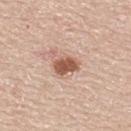The tile uses white-light illumination.
The lesion is located on the upper back.
An algorithmic analysis of the crop reported an area of roughly 5.5 mm² and a shape eccentricity near 0.75. And it measured a border-irregularity index near 2/10, a color-variation rating of about 2.5/10, and a peripheral color-asymmetry measure near 1.
A male subject, about 60 years old.
Longest diameter approximately 3 mm.
A lesion tile, about 15 mm wide, cut from a 3D total-body photograph.